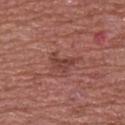follow-up: catalogued during a skin exam; not biopsied
diameter: ≈3 mm
anatomic site: the upper back
image: total-body-photography crop, ~15 mm field of view
lighting: white-light
subject: male, aged 53–57